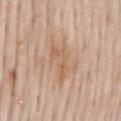No biopsy was performed on this lesion — it was imaged during a full skin examination and was not determined to be concerning. Located on the mid back. Imaged with white-light lighting. A region of skin cropped from a whole-body photographic capture, roughly 15 mm wide. A male subject in their mid-60s. The lesion's longest dimension is about 4.5 mm.